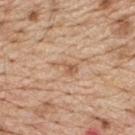Assessment:
This lesion was catalogued during total-body skin photography and was not selected for biopsy.
Clinical summary:
From the upper back. The lesion's longest dimension is about 3 mm. Imaged with white-light lighting. The lesion-visualizer software estimated a lesion color around L≈59 a*≈20 b*≈34 in CIELAB, a lesion–skin lightness drop of about 8, and a lesion-to-skin contrast of about 6 (normalized; higher = more distinct). The software also gave border irregularity of about 6 on a 0–10 scale, a color-variation rating of about 1.5/10, and radial color variation of about 0.5. The software also gave an automated nevus-likeness rating near 0 out of 100 and a detector confidence of about 100 out of 100 that the crop contains a lesion. A region of skin cropped from a whole-body photographic capture, roughly 15 mm wide. A male subject aged 68–72.